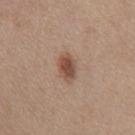This lesion was catalogued during total-body skin photography and was not selected for biopsy. A 15 mm crop from a total-body photograph taken for skin-cancer surveillance. The tile uses white-light illumination. Located on the chest. A female patient in their mid- to late 40s. Automated image analysis of the tile measured a classifier nevus-likeness of about 95/100 and lesion-presence confidence of about 100/100.